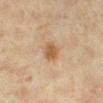Assessment:
This lesion was catalogued during total-body skin photography and was not selected for biopsy.
Acquisition and patient details:
About 3 mm across. A 15 mm crop from a total-body photograph taken for skin-cancer surveillance. Located on the leg. The tile uses cross-polarized illumination. A female patient, about 40 years old.